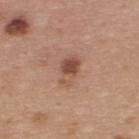The lesion was photographed on a routine skin check and not biopsied; there is no pathology result.
The subject is a male about 35 years old.
An algorithmic analysis of the crop reported roughly 11 lightness units darker than nearby skin and a normalized lesion–skin contrast near 7.5. It also reported a border-irregularity index near 3/10, internal color variation of about 4.5 on a 0–10 scale, and a peripheral color-asymmetry measure near 1.5.
Captured under white-light illumination.
Measured at roughly 3.5 mm in maximum diameter.
The lesion is located on the back.
Cropped from a whole-body photographic skin survey; the tile spans about 15 mm.